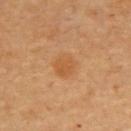| feature | finding |
|---|---|
| diameter | about 3 mm |
| acquisition | ~15 mm tile from a whole-body skin photo |
| site | the upper back |
| subject | approximately 60 years of age |
| tile lighting | cross-polarized |